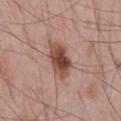No biopsy was performed on this lesion — it was imaged during a full skin examination and was not determined to be concerning.
Cropped from a whole-body photographic skin survey; the tile spans about 15 mm.
The subject is a male aged 43 to 47.
Located on the mid back.
The total-body-photography lesion software estimated about 15 CIELAB-L* units darker than the surrounding skin and a normalized lesion–skin contrast near 10.5. The software also gave a border-irregularity rating of about 2.5/10, internal color variation of about 6 on a 0–10 scale, and a peripheral color-asymmetry measure near 1.5. It also reported an automated nevus-likeness rating near 95 out of 100 and a detector confidence of about 100 out of 100 that the crop contains a lesion.
The tile uses white-light illumination.